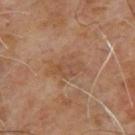biopsy status = total-body-photography surveillance lesion; no biopsy
patient = male, approximately 65 years of age
anatomic site = the upper back
image = total-body-photography crop, ~15 mm field of view